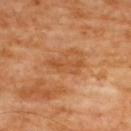notes=no biopsy performed (imaged during a skin exam) | imaging modality=~15 mm tile from a whole-body skin photo | body site=the upper back | patient=female, roughly 65 years of age | illumination=cross-polarized illumination | diameter=~4.5 mm (longest diameter).